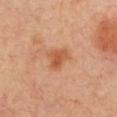No biopsy was performed on this lesion — it was imaged during a full skin examination and was not determined to be concerning. A close-up tile cropped from a whole-body skin photograph, about 15 mm across. Automated image analysis of the tile measured a lesion–skin lightness drop of about 9. And it measured a nevus-likeness score of about 55/100. This is a cross-polarized tile. The lesion is located on the chest. A female patient aged approximately 40.